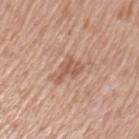The lesion's longest dimension is about 3.5 mm. A female subject aged 48 to 52. A close-up tile cropped from a whole-body skin photograph, about 15 mm across. The tile uses white-light illumination. On the left upper arm.